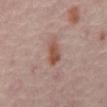Imaged during a routine full-body skin examination; the lesion was not biopsied and no histopathology is available. The patient is in their mid- to late 50s. The total-body-photography lesion software estimated an area of roughly 5.5 mm², an outline eccentricity of about 0.9 (0 = round, 1 = elongated), and a shape-asymmetry score of about 0.25 (0 = symmetric). The analysis additionally found border irregularity of about 2.5 on a 0–10 scale, a within-lesion color-variation index near 3/10, and peripheral color asymmetry of about 1. The lesion is located on the front of the torso. A lesion tile, about 15 mm wide, cut from a 3D total-body photograph. The tile uses cross-polarized illumination.